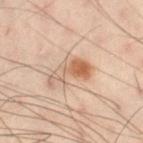Impression: No biopsy was performed on this lesion — it was imaged during a full skin examination and was not determined to be concerning. Background: The total-body-photography lesion software estimated a lesion area of about 8.5 mm² and an eccentricity of roughly 0.85. The analysis additionally found a lesion color around L≈50 a*≈16 b*≈27 in CIELAB, a lesion–skin lightness drop of about 9, and a normalized lesion–skin contrast near 7. It also reported a border-irregularity index near 6/10, a within-lesion color-variation index near 7.5/10, and a peripheral color-asymmetry measure near 2.5. And it measured lesion-presence confidence of about 100/100. A male subject, aged around 50. The lesion's longest dimension is about 4.5 mm. This image is a 15 mm lesion crop taken from a total-body photograph. The lesion is on the left leg.